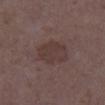biopsy status = catalogued during a skin exam; not biopsied | imaging modality = ~15 mm tile from a whole-body skin photo | body site = the right lower leg | patient = female, in their mid- to late 30s | illumination = white-light illumination | lesion diameter = about 4.5 mm.